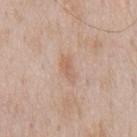Clinical impression:
No biopsy was performed on this lesion — it was imaged during a full skin examination and was not determined to be concerning.
Clinical summary:
A roughly 15 mm field-of-view crop from a total-body skin photograph. Imaged with white-light lighting. Automated tile analysis of the lesion measured an average lesion color of about L≈62 a*≈18 b*≈30 (CIELAB), a lesion–skin lightness drop of about 7, and a normalized border contrast of about 5.5. The subject is a male about 60 years old. About 3 mm across. Located on the chest.